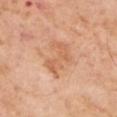follow-up: catalogued during a skin exam; not biopsied
subject: male, in their mid- to late 50s
diameter: ≈5 mm
anatomic site: the left upper arm
TBP lesion metrics: a lesion area of about 8.5 mm² and an outline eccentricity of about 0.85 (0 = round, 1 = elongated); an average lesion color of about L≈62 a*≈23 b*≈36 (CIELAB), a lesion–skin lightness drop of about 8, and a normalized border contrast of about 5.5; an automated nevus-likeness rating near 0 out of 100 and a lesion-detection confidence of about 100/100
acquisition: total-body-photography crop, ~15 mm field of view
lighting: cross-polarized illumination